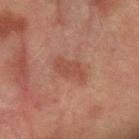<lesion>
<biopsy_status>not biopsied; imaged during a skin examination</biopsy_status>
<image>
  <source>total-body photography crop</source>
  <field_of_view_mm>15</field_of_view_mm>
</image>
<lighting>cross-polarized</lighting>
<site>left forearm</site>
<automated_metrics>
  <nevus_likeness_0_100>10</nevus_likeness_0_100>
</automated_metrics>
<patient>
  <sex>male</sex>
  <age_approx>75</age_approx>
</patient>
</lesion>